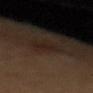acquisition = 15 mm crop, total-body photography; subject = female, roughly 40 years of age; location = the upper back; size = ≈3 mm.